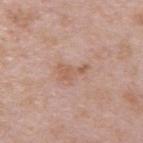Q: Was a biopsy performed?
A: imaged on a skin check; not biopsied
Q: Patient demographics?
A: male, roughly 40 years of age
Q: Lesion location?
A: the upper back
Q: How was this image acquired?
A: total-body-photography crop, ~15 mm field of view